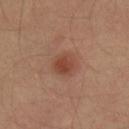Imaged during a routine full-body skin examination; the lesion was not biopsied and no histopathology is available. On the right thigh. A region of skin cropped from a whole-body photographic capture, roughly 15 mm wide. A male patient in their 40s. Measured at roughly 2.5 mm in maximum diameter.